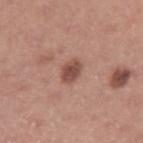Recorded during total-body skin imaging; not selected for excision or biopsy.
This image is a 15 mm lesion crop taken from a total-body photograph.
Imaged with white-light lighting.
The lesion is on the left upper arm.
The lesion's longest dimension is about 3 mm.
A male patient, roughly 40 years of age.